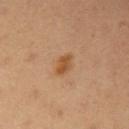<lesion>
  <biopsy_status>not biopsied; imaged during a skin examination</biopsy_status>
  <lighting>cross-polarized</lighting>
  <lesion_size>
    <long_diameter_mm_approx>2.5</long_diameter_mm_approx>
  </lesion_size>
  <patient>
    <sex>female</sex>
    <age_approx>35</age_approx>
  </patient>
  <image>
    <source>total-body photography crop</source>
    <field_of_view_mm>15</field_of_view_mm>
  </image>
  <site>right upper arm</site>
</lesion>